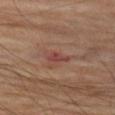This lesion was catalogued during total-body skin photography and was not selected for biopsy. The total-body-photography lesion software estimated a border-irregularity rating of about 3/10 and internal color variation of about 0.5 on a 0–10 scale. The software also gave an automated nevus-likeness rating near 0 out of 100 and a lesion-detection confidence of about 100/100. A male subject, in their 70s. A region of skin cropped from a whole-body photographic capture, roughly 15 mm wide. This is a cross-polarized tile. On the left thigh.